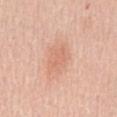Impression: The lesion was photographed on a routine skin check and not biopsied; there is no pathology result. Image and clinical context: The lesion-visualizer software estimated border irregularity of about 3 on a 0–10 scale and a within-lesion color-variation index near 2/10. And it measured a lesion-detection confidence of about 100/100. The tile uses white-light illumination. Approximately 4 mm at its widest. A region of skin cropped from a whole-body photographic capture, roughly 15 mm wide. On the mid back. A female subject, aged 63–67.